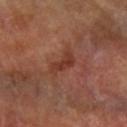Imaged during a routine full-body skin examination; the lesion was not biopsied and no histopathology is available. A 15 mm crop from a total-body photograph taken for skin-cancer surveillance. A female patient, about 60 years old. From the left arm. The recorded lesion diameter is about 3.5 mm. This is a cross-polarized tile.